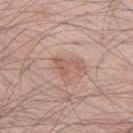Recorded during total-body skin imaging; not selected for excision or biopsy. A male subject roughly 60 years of age. Approximately 3.5 mm at its widest. A 15 mm close-up tile from a total-body photography series done for melanoma screening. Imaged with white-light lighting. Located on the leg.